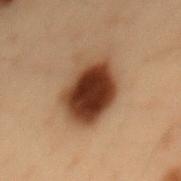Q: Was this lesion biopsied?
A: catalogued during a skin exam; not biopsied
Q: Where on the body is the lesion?
A: the mid back
Q: Who is the patient?
A: male, roughly 55 years of age
Q: How was the tile lit?
A: cross-polarized illumination
Q: What is the imaging modality?
A: 15 mm crop, total-body photography
Q: How large is the lesion?
A: ≈7 mm